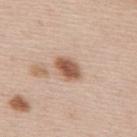This lesion was catalogued during total-body skin photography and was not selected for biopsy.
The recorded lesion diameter is about 3.5 mm.
The subject is a male aged 43 to 47.
The lesion is located on the mid back.
A lesion tile, about 15 mm wide, cut from a 3D total-body photograph.
The total-body-photography lesion software estimated an area of roughly 6 mm², an eccentricity of roughly 0.75, and a shape-asymmetry score of about 0.2 (0 = symmetric). The analysis additionally found a lesion–skin lightness drop of about 15 and a normalized lesion–skin contrast near 10. And it measured a color-variation rating of about 4/10 and radial color variation of about 1. It also reported a nevus-likeness score of about 95/100 and a detector confidence of about 100 out of 100 that the crop contains a lesion.